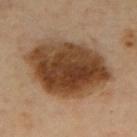biopsy status: total-body-photography surveillance lesion; no biopsy
TBP lesion metrics: a nevus-likeness score of about 65/100 and a detector confidence of about 100 out of 100 that the crop contains a lesion
image source: 15 mm crop, total-body photography
anatomic site: the upper back
subject: male, aged 63–67
size: ~10 mm (longest diameter)